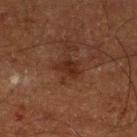Q: Was this lesion biopsied?
A: total-body-photography surveillance lesion; no biopsy
Q: What is the lesion's diameter?
A: ≈2.5 mm
Q: What is the imaging modality?
A: ~15 mm crop, total-body skin-cancer survey
Q: Lesion location?
A: the left lower leg
Q: Who is the patient?
A: male, aged approximately 65
Q: How was the tile lit?
A: cross-polarized